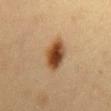follow-up = no biopsy performed (imaged during a skin exam)
anatomic site = the chest
imaging modality = total-body-photography crop, ~15 mm field of view
subject = female, roughly 30 years of age